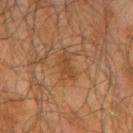Assessment: Recorded during total-body skin imaging; not selected for excision or biopsy. Clinical summary: A male patient in their mid-60s. Cropped from a whole-body photographic skin survey; the tile spans about 15 mm. Longest diameter approximately 3.5 mm. Located on the arm.